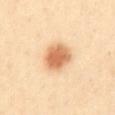Part of a total-body skin-imaging series; this lesion was reviewed on a skin check and was not flagged for biopsy.
On the mid back.
The subject is a female aged 38 to 42.
This image is a 15 mm lesion crop taken from a total-body photograph.